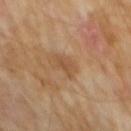workup: imaged on a skin check; not biopsied | subject: male, roughly 60 years of age | image source: ~15 mm tile from a whole-body skin photo | automated metrics: border irregularity of about 3.5 on a 0–10 scale and a within-lesion color-variation index near 1.5/10 | diameter: ~3 mm (longest diameter) | anatomic site: the abdomen | lighting: cross-polarized.